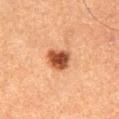Part of a total-body skin-imaging series; this lesion was reviewed on a skin check and was not flagged for biopsy. Imaged with cross-polarized lighting. The recorded lesion diameter is about 3 mm. An algorithmic analysis of the crop reported an area of roughly 7.5 mm², an outline eccentricity of about 0.55 (0 = round, 1 = elongated), and a symmetry-axis asymmetry near 0.25. The analysis additionally found a lesion color around L≈42 a*≈24 b*≈32 in CIELAB, roughly 16 lightness units darker than nearby skin, and a lesion-to-skin contrast of about 11.5 (normalized; higher = more distinct). The software also gave a classifier nevus-likeness of about 100/100 and a detector confidence of about 100 out of 100 that the crop contains a lesion. A region of skin cropped from a whole-body photographic capture, roughly 15 mm wide. A female patient, aged around 60. The lesion is located on the right thigh.